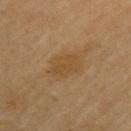Q: Lesion location?
A: the arm
Q: What kind of image is this?
A: total-body-photography crop, ~15 mm field of view
Q: What is the lesion's diameter?
A: ~4 mm (longest diameter)
Q: What did automated image analysis measure?
A: a mean CIELAB color near L≈44 a*≈16 b*≈34 and a lesion-to-skin contrast of about 5.5 (normalized; higher = more distinct); internal color variation of about 1.5 on a 0–10 scale and a peripheral color-asymmetry measure near 0.5; a classifier nevus-likeness of about 15/100 and a lesion-detection confidence of about 100/100
Q: Who is the patient?
A: male, roughly 70 years of age
Q: What lighting was used for the tile?
A: cross-polarized illumination